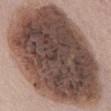Captured during whole-body skin photography for melanoma surveillance; the lesion was not biopsied. Measured at roughly 15.5 mm in maximum diameter. This image is a 15 mm lesion crop taken from a total-body photograph. An algorithmic analysis of the crop reported roughly 21 lightness units darker than nearby skin. Imaged with white-light lighting. The subject is a male aged approximately 75. The lesion is located on the abdomen.